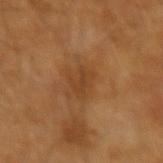<tbp_lesion>
  <biopsy_status>not biopsied; imaged during a skin examination</biopsy_status>
  <lesion_size>
    <long_diameter_mm_approx>3.0</long_diameter_mm_approx>
  </lesion_size>
  <automated_metrics>
    <area_mm2_approx>4.5</area_mm2_approx>
    <eccentricity>0.75</eccentricity>
    <shape_asymmetry>0.45</shape_asymmetry>
    <border_irregularity_0_10>5.5</border_irregularity_0_10>
    <peripheral_color_asymmetry>0.5</peripheral_color_asymmetry>
    <nevus_likeness_0_100>5</nevus_likeness_0_100>
    <lesion_detection_confidence_0_100>100</lesion_detection_confidence_0_100>
  </automated_metrics>
  <lighting>cross-polarized</lighting>
  <patient>
    <sex>male</sex>
    <age_approx>65</age_approx>
  </patient>
  <image>
    <source>total-body photography crop</source>
    <field_of_view_mm>15</field_of_view_mm>
  </image>
</tbp_lesion>